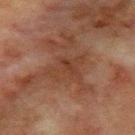Q: Was this lesion biopsied?
A: total-body-photography surveillance lesion; no biopsy
Q: What is the lesion's diameter?
A: ~4 mm (longest diameter)
Q: What kind of image is this?
A: total-body-photography crop, ~15 mm field of view
Q: Lesion location?
A: the chest
Q: How was the tile lit?
A: cross-polarized
Q: Automated lesion metrics?
A: roughly 5 lightness units darker than nearby skin and a normalized lesion–skin contrast near 5.5; an automated nevus-likeness rating near 0 out of 100
Q: What are the patient's age and sex?
A: male, aged 63 to 67